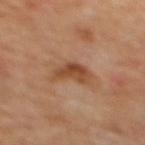<record>
  <biopsy_status>not biopsied; imaged during a skin examination</biopsy_status>
  <site>mid back</site>
  <patient>
    <age_approx>65</age_approx>
  </patient>
  <image>
    <source>total-body photography crop</source>
    <field_of_view_mm>15</field_of_view_mm>
  </image>
  <lesion_size>
    <long_diameter_mm_approx>3.5</long_diameter_mm_approx>
  </lesion_size>
  <lighting>cross-polarized</lighting>
</record>